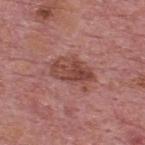Q: What is the anatomic site?
A: the upper back
Q: What is the imaging modality?
A: 15 mm crop, total-body photography
Q: Patient demographics?
A: male, in their mid- to late 70s